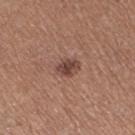| field | value |
|---|---|
| notes | catalogued during a skin exam; not biopsied |
| subject | female, aged 33–37 |
| lesion size | ~3 mm (longest diameter) |
| automated metrics | a footprint of about 5 mm², a shape eccentricity near 0.6, and a symmetry-axis asymmetry near 0.15; a lesion color around L≈44 a*≈20 b*≈24 in CIELAB, roughly 11 lightness units darker than nearby skin, and a normalized border contrast of about 8.5; a border-irregularity index near 1.5/10 and internal color variation of about 3 on a 0–10 scale; lesion-presence confidence of about 100/100 |
| image source | 15 mm crop, total-body photography |
| tile lighting | white-light illumination |
| anatomic site | the leg |